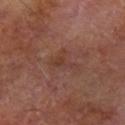No biopsy was performed on this lesion — it was imaged during a full skin examination and was not determined to be concerning. Automated image analysis of the tile measured a footprint of about 4 mm², an outline eccentricity of about 0.8 (0 = round, 1 = elongated), and a shape-asymmetry score of about 0.6 (0 = symmetric). The software also gave a mean CIELAB color near L≈37 a*≈20 b*≈25, roughly 6 lightness units darker than nearby skin, and a normalized lesion–skin contrast near 5.5. The software also gave a classifier nevus-likeness of about 0/100 and a detector confidence of about 100 out of 100 that the crop contains a lesion. Approximately 3.5 mm at its widest. The lesion is located on the left forearm. A 15 mm close-up tile from a total-body photography series done for melanoma screening. A male subject, aged approximately 70.